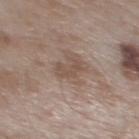Assessment:
Captured during whole-body skin photography for melanoma surveillance; the lesion was not biopsied.
Acquisition and patient details:
A 15 mm close-up tile from a total-body photography series done for melanoma screening. The lesion is on the back. The patient is a male in their mid- to late 50s.